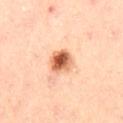No biopsy was performed on this lesion — it was imaged during a full skin examination and was not determined to be concerning. Cropped from a total-body skin-imaging series; the visible field is about 15 mm. Located on the right thigh. A female patient, in their mid- to late 40s. Automated image analysis of the tile measured an area of roughly 7 mm², a shape eccentricity near 0.35, and two-axis asymmetry of about 0.15. And it measured a lesion color around L≈62 a*≈26 b*≈37 in CIELAB and a normalized border contrast of about 11. And it measured border irregularity of about 1.5 on a 0–10 scale, a within-lesion color-variation index near 9.5/10, and a peripheral color-asymmetry measure near 3.